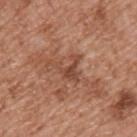biopsy status = no biopsy performed (imaged during a skin exam)
location = the upper back
TBP lesion metrics = a classifier nevus-likeness of about 0/100 and a lesion-detection confidence of about 100/100
subject = male, in their mid-50s
size = ≈4 mm
image = total-body-photography crop, ~15 mm field of view